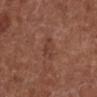Impression: Recorded during total-body skin imaging; not selected for excision or biopsy. Context: A 15 mm close-up tile from a total-body photography series done for melanoma screening. About 3 mm across. The tile uses white-light illumination. From the left lower leg. A male patient in their mid-70s.